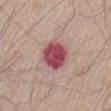workup: no biopsy performed (imaged during a skin exam)
location: the lower back
image source: ~15 mm tile from a whole-body skin photo
patient: male, about 65 years old